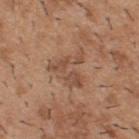site: the upper back
subject: male, aged approximately 40
imaging modality: ~15 mm crop, total-body skin-cancer survey
automated metrics: a lesion color around L≈50 a*≈20 b*≈30 in CIELAB and a normalized lesion–skin contrast near 6; border irregularity of about 9.5 on a 0–10 scale, a color-variation rating of about 2.5/10, and a peripheral color-asymmetry measure near 1
tile lighting: white-light
diameter: about 5 mm